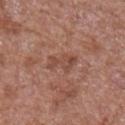Impression: The lesion was photographed on a routine skin check and not biopsied; there is no pathology result. Background: A male subject, in their mid-60s. A 15 mm close-up tile from a total-body photography series done for melanoma screening. Automated tile analysis of the lesion measured a border-irregularity rating of about 5/10, internal color variation of about 3.5 on a 0–10 scale, and radial color variation of about 1. The lesion's longest dimension is about 4 mm. From the right upper arm.